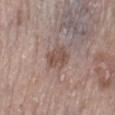Imaged during a routine full-body skin examination; the lesion was not biopsied and no histopathology is available. The subject is a female in their mid- to late 60s. This image is a 15 mm lesion crop taken from a total-body photograph. The lesion's longest dimension is about 3.5 mm. On the left lower leg.